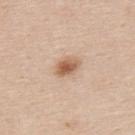Q: Is there a histopathology result?
A: imaged on a skin check; not biopsied
Q: Lesion location?
A: the back
Q: What are the patient's age and sex?
A: male, approximately 45 years of age
Q: What is the imaging modality?
A: ~15 mm tile from a whole-body skin photo
Q: Lesion size?
A: ≈3 mm
Q: Illumination type?
A: white-light illumination